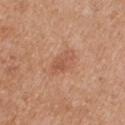Background:
The subject is a male in their mid-50s. On the right upper arm. This image is a 15 mm lesion crop taken from a total-body photograph.